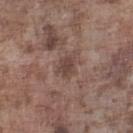Clinical impression: Recorded during total-body skin imaging; not selected for excision or biopsy. Image and clinical context: Located on the leg. Longest diameter approximately 3 mm. A region of skin cropped from a whole-body photographic capture, roughly 15 mm wide. The subject is a male in their mid-70s. The tile uses white-light illumination.